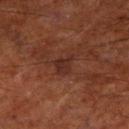tile lighting — cross-polarized illumination | automated lesion analysis — an outline eccentricity of about 0.65 (0 = round, 1 = elongated) and a shape-asymmetry score of about 0.4 (0 = symmetric); a lesion color around L≈27 a*≈22 b*≈23 in CIELAB, roughly 6 lightness units darker than nearby skin, and a lesion-to-skin contrast of about 7 (normalized; higher = more distinct); an automated nevus-likeness rating near 0 out of 100 and a lesion-detection confidence of about 100/100 | anatomic site — the left lower leg | image — ~15 mm tile from a whole-body skin photo | size — ~2.5 mm (longest diameter) | subject — male, approximately 65 years of age.